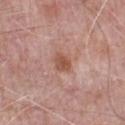Impression:
Recorded during total-body skin imaging; not selected for excision or biopsy.
Image and clinical context:
Approximately 2.5 mm at its widest. Located on the front of the torso. This is a white-light tile. A lesion tile, about 15 mm wide, cut from a 3D total-body photograph. The subject is a male about 70 years old.